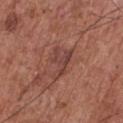No biopsy was performed on this lesion — it was imaged during a full skin examination and was not determined to be concerning. From the chest. A roughly 15 mm field-of-view crop from a total-body skin photograph. The tile uses white-light illumination. The subject is a male aged 73 to 77.